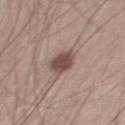Part of a total-body skin-imaging series; this lesion was reviewed on a skin check and was not flagged for biopsy.
On the right thigh.
A lesion tile, about 15 mm wide, cut from a 3D total-body photograph.
A male subject aged approximately 60.
Automated tile analysis of the lesion measured an average lesion color of about L≈48 a*≈18 b*≈23 (CIELAB), roughly 13 lightness units darker than nearby skin, and a lesion-to-skin contrast of about 9.5 (normalized; higher = more distinct). The software also gave a color-variation rating of about 1.5/10 and peripheral color asymmetry of about 0.5. It also reported a classifier nevus-likeness of about 90/100 and a detector confidence of about 100 out of 100 that the crop contains a lesion.
This is a white-light tile.